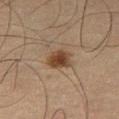Impression: This lesion was catalogued during total-body skin photography and was not selected for biopsy. Context: The lesion-visualizer software estimated an area of roughly 6 mm², an eccentricity of roughly 0.5, and a shape-asymmetry score of about 0.25 (0 = symmetric). And it measured an average lesion color of about L≈34 a*≈15 b*≈26 (CIELAB) and a normalized border contrast of about 10. It also reported internal color variation of about 3 on a 0–10 scale and peripheral color asymmetry of about 0.5. It also reported a classifier nevus-likeness of about 100/100 and a lesion-detection confidence of about 100/100. A male subject, in their mid-60s. About 3 mm across. This is a cross-polarized tile. A 15 mm close-up tile from a total-body photography series done for melanoma screening. The lesion is on the right thigh.